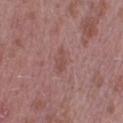The lesion was tiled from a total-body skin photograph and was not biopsied.
A 15 mm close-up extracted from a 3D total-body photography capture.
Longest diameter approximately 2.5 mm.
This is a white-light tile.
Located on the right lower leg.
A male patient, aged 38 to 42.